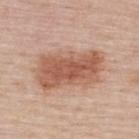No biopsy was performed on this lesion — it was imaged during a full skin examination and was not determined to be concerning. Cropped from a total-body skin-imaging series; the visible field is about 15 mm. Approximately 7.5 mm at its widest. From the upper back. The subject is a female about 50 years old. The tile uses white-light illumination.